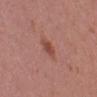No biopsy was performed on this lesion — it was imaged during a full skin examination and was not determined to be concerning. A 15 mm close-up extracted from a 3D total-body photography capture. The patient is a female about 15 years old. The lesion is on the left thigh.